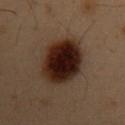The lesion was photographed on a routine skin check and not biopsied; there is no pathology result. A roughly 15 mm field-of-view crop from a total-body skin photograph. The lesion is located on the mid back. A male patient, in their mid- to late 50s.